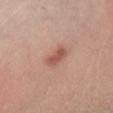This lesion was catalogued during total-body skin photography and was not selected for biopsy. A male subject about 40 years old. Imaged with cross-polarized lighting. The lesion is on the right lower leg. A lesion tile, about 15 mm wide, cut from a 3D total-body photograph. Automated image analysis of the tile measured an area of roughly 4.5 mm² and a shape eccentricity near 0.8. The analysis additionally found a border-irregularity index near 2/10 and radial color variation of about 0.5. It also reported an automated nevus-likeness rating near 90 out of 100. The lesion's longest dimension is about 3 mm.